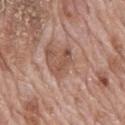Clinical impression:
No biopsy was performed on this lesion — it was imaged during a full skin examination and was not determined to be concerning.
Image and clinical context:
A male subject approximately 70 years of age. Captured under white-light illumination. Cropped from a total-body skin-imaging series; the visible field is about 15 mm. Automated tile analysis of the lesion measured border irregularity of about 3.5 on a 0–10 scale and radial color variation of about 0.5. About 3.5 mm across. The lesion is on the mid back.